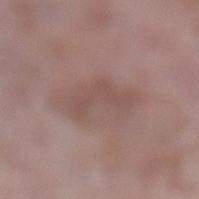No biopsy was performed on this lesion — it was imaged during a full skin examination and was not determined to be concerning. Captured under white-light illumination. From the leg. The recorded lesion diameter is about 5 mm. A female patient aged approximately 70. A lesion tile, about 15 mm wide, cut from a 3D total-body photograph. The total-body-photography lesion software estimated a lesion color around L≈50 a*≈18 b*≈22 in CIELAB, about 6 CIELAB-L* units darker than the surrounding skin, and a normalized lesion–skin contrast near 5. The software also gave border irregularity of about 7 on a 0–10 scale, a color-variation rating of about 1.5/10, and radial color variation of about 0.5. It also reported a nevus-likeness score of about 0/100 and a detector confidence of about 100 out of 100 that the crop contains a lesion.